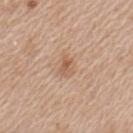– biopsy status · catalogued during a skin exam; not biopsied
– anatomic site · the left upper arm
– lighting · white-light
– image · total-body-photography crop, ~15 mm field of view
– diameter · ≈2.5 mm
– patient · female, about 40 years old